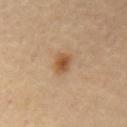The lesion was photographed on a routine skin check and not biopsied; there is no pathology result.
A 15 mm crop from a total-body photograph taken for skin-cancer surveillance.
Located on the mid back.
A male patient, approximately 70 years of age.
Automated image analysis of the tile measured a lesion area of about 4.5 mm², a shape eccentricity near 0.75, and a shape-asymmetry score of about 0.25 (0 = symmetric). The analysis additionally found a within-lesion color-variation index near 4/10 and peripheral color asymmetry of about 1.5.
About 3 mm across.